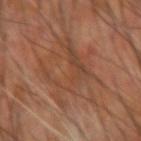  biopsy_status: not biopsied; imaged during a skin examination